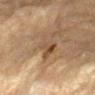Q: Is there a histopathology result?
A: catalogued during a skin exam; not biopsied
Q: Patient demographics?
A: male, in their mid- to late 80s or older
Q: How was this image acquired?
A: ~15 mm crop, total-body skin-cancer survey
Q: What is the anatomic site?
A: the left forearm